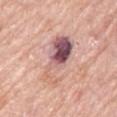Notes:
- biopsy status: catalogued during a skin exam; not biopsied
- imaging modality: ~15 mm crop, total-body skin-cancer survey
- subject: female, aged 63–67
- anatomic site: the right upper arm